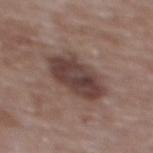follow-up: total-body-photography surveillance lesion; no biopsy | patient: female, aged around 65 | tile lighting: white-light | image-analysis metrics: a footprint of about 18 mm², an eccentricity of roughly 0.85, and a symmetry-axis asymmetry near 0.2; a mean CIELAB color near L≈40 a*≈17 b*≈20, roughly 12 lightness units darker than nearby skin, and a lesion-to-skin contrast of about 10 (normalized; higher = more distinct); a border-irregularity rating of about 2.5/10 and peripheral color asymmetry of about 1.5 | diameter: ~6.5 mm (longest diameter) | image source: 15 mm crop, total-body photography | anatomic site: the back.